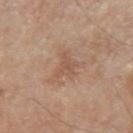The lesion was photographed on a routine skin check and not biopsied; there is no pathology result. A male subject, roughly 80 years of age. The tile uses white-light illumination. Longest diameter approximately 3 mm. This image is a 15 mm lesion crop taken from a total-body photograph. Located on the left upper arm.